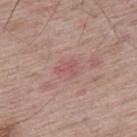biopsy_status: not biopsied; imaged during a skin examination
site: upper back
image:
  source: total-body photography crop
  field_of_view_mm: 15
patient:
  sex: male
  age_approx: 65
lighting: white-light